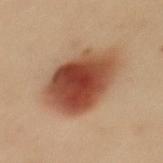Q: Was a biopsy performed?
A: no biopsy performed (imaged during a skin exam)
Q: Patient demographics?
A: female, aged 33–37
Q: What is the anatomic site?
A: the mid back
Q: What lighting was used for the tile?
A: cross-polarized illumination
Q: What kind of image is this?
A: ~15 mm tile from a whole-body skin photo
Q: How large is the lesion?
A: ≈7.5 mm